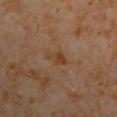Recorded during total-body skin imaging; not selected for excision or biopsy.
Located on the left upper arm.
A region of skin cropped from a whole-body photographic capture, roughly 15 mm wide.
The lesion-visualizer software estimated an outline eccentricity of about 0.7 (0 = round, 1 = elongated) and two-axis asymmetry of about 0.4. The analysis additionally found a peripheral color-asymmetry measure near 0.5.
A male patient, approximately 60 years of age.
Imaged with cross-polarized lighting.
Longest diameter approximately 2.5 mm.